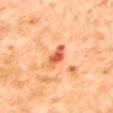Captured during whole-body skin photography for melanoma surveillance; the lesion was not biopsied. A lesion tile, about 15 mm wide, cut from a 3D total-body photograph. The lesion is located on the upper back. A female patient about 55 years old. Automated image analysis of the tile measured an average lesion color of about L≈65 a*≈39 b*≈44 (CIELAB), about 15 CIELAB-L* units darker than the surrounding skin, and a normalized lesion–skin contrast near 8.5. It also reported a border-irregularity index near 4/10 and a color-variation rating of about 3.5/10. Captured under cross-polarized illumination. The recorded lesion diameter is about 4 mm.